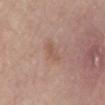Assessment: The lesion was photographed on a routine skin check and not biopsied; there is no pathology result. Background: A male patient, aged 78 to 82. An algorithmic analysis of the crop reported a footprint of about 4 mm², an eccentricity of roughly 0.9, and two-axis asymmetry of about 0.3. The software also gave a mean CIELAB color near L≈55 a*≈18 b*≈27, about 6 CIELAB-L* units darker than the surrounding skin, and a normalized lesion–skin contrast near 4.5. It also reported border irregularity of about 3.5 on a 0–10 scale and internal color variation of about 1.5 on a 0–10 scale. The analysis additionally found a lesion-detection confidence of about 100/100. Captured under white-light illumination. Cropped from a whole-body photographic skin survey; the tile spans about 15 mm. The lesion is located on the mid back.